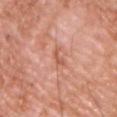Impression:
The lesion was photographed on a routine skin check and not biopsied; there is no pathology result.
Acquisition and patient details:
Longest diameter approximately 3 mm. The lesion-visualizer software estimated a footprint of about 2.5 mm² and an outline eccentricity of about 0.95 (0 = round, 1 = elongated). The software also gave an automated nevus-likeness rating near 0 out of 100. The patient is a male aged around 60. Located on the front of the torso. A roughly 15 mm field-of-view crop from a total-body skin photograph.